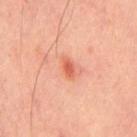site = the mid back
subject = male, aged 43–47
automated metrics = a lesion area of about 3 mm² and a shape eccentricity near 0.8; a border-irregularity index near 2/10, a within-lesion color-variation index near 2/10, and peripheral color asymmetry of about 0.5
imaging modality = ~15 mm crop, total-body skin-cancer survey
lesion size = ~2.5 mm (longest diameter)
lighting = cross-polarized